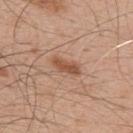notes — imaged on a skin check; not biopsied
tile lighting — white-light illumination
acquisition — ~15 mm crop, total-body skin-cancer survey
subject — male, in their 50s
location — the upper back
diameter — ≈3.5 mm